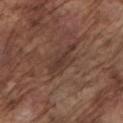biopsy status: catalogued during a skin exam; not biopsied
imaging modality: ~15 mm crop, total-body skin-cancer survey
patient: male, roughly 75 years of age
lesion diameter: ≈5 mm
lighting: white-light
site: the left upper arm